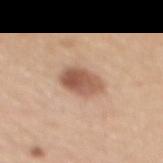workup: imaged on a skin check; not biopsied
tile lighting: white-light
acquisition: ~15 mm crop, total-body skin-cancer survey
diameter: about 4 mm
site: the mid back
patient: female, in their mid- to late 50s
image-analysis metrics: a footprint of about 9 mm², an outline eccentricity of about 0.75 (0 = round, 1 = elongated), and a shape-asymmetry score of about 0.15 (0 = symmetric); a mean CIELAB color near L≈56 a*≈21 b*≈30 and a normalized border contrast of about 9; a color-variation rating of about 6/10 and a peripheral color-asymmetry measure near 2.5; an automated nevus-likeness rating near 95 out of 100 and a detector confidence of about 100 out of 100 that the crop contains a lesion